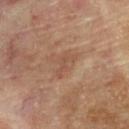biopsy status = no biopsy performed (imaged during a skin exam)
subject = male, roughly 85 years of age or older
site = the upper back
size = ≈3.5 mm
automated lesion analysis = an area of roughly 3.5 mm², an eccentricity of roughly 0.9, and a symmetry-axis asymmetry near 0.55; an average lesion color of about L≈49 a*≈20 b*≈28 (CIELAB), roughly 6 lightness units darker than nearby skin, and a normalized lesion–skin contrast near 4.5; a border-irregularity rating of about 6.5/10, a color-variation rating of about 0/10, and peripheral color asymmetry of about 0; lesion-presence confidence of about 100/100
acquisition = 15 mm crop, total-body photography
illumination = cross-polarized